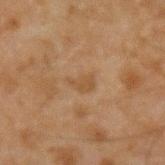Impression:
The lesion was tiled from a total-body skin photograph and was not biopsied.